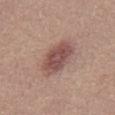This lesion was catalogued during total-body skin photography and was not selected for biopsy. From the abdomen. The patient is a male aged 23–27. A lesion tile, about 15 mm wide, cut from a 3D total-body photograph.